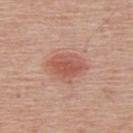Notes:
* workup — imaged on a skin check; not biopsied
* anatomic site — the back
* image source — ~15 mm tile from a whole-body skin photo
* subject — male, aged around 60